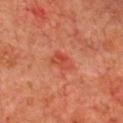This is a cross-polarized tile. Longest diameter approximately 2.5 mm. This image is a 15 mm lesion crop taken from a total-body photograph. A male subject, aged approximately 70. Located on the chest.